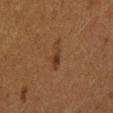biopsy status: imaged on a skin check; not biopsied | imaging modality: total-body-photography crop, ~15 mm field of view | TBP lesion metrics: a lesion area of about 4 mm², an eccentricity of roughly 0.95, and two-axis asymmetry of about 0.35; a mean CIELAB color near L≈29 a*≈17 b*≈26, a lesion–skin lightness drop of about 6, and a lesion-to-skin contrast of about 6.5 (normalized; higher = more distinct); a border-irregularity index near 4.5/10, a within-lesion color-variation index near 1.5/10, and a peripheral color-asymmetry measure near 0.5; a nevus-likeness score of about 35/100 and a lesion-detection confidence of about 100/100 | subject: female, aged approximately 50 | lighting: cross-polarized | lesion size: ≈3.5 mm | anatomic site: the right thigh.